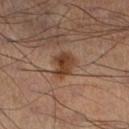Q: Was a biopsy performed?
A: no biopsy performed (imaged during a skin exam)